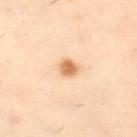A female subject, aged 43–47. Located on the right thigh. A lesion tile, about 15 mm wide, cut from a 3D total-body photograph.